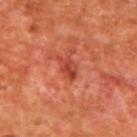No biopsy was performed on this lesion — it was imaged during a full skin examination and was not determined to be concerning. Cropped from a whole-body photographic skin survey; the tile spans about 15 mm. The subject is a male aged 63–67. An algorithmic analysis of the crop reported a shape eccentricity near 0.8. It also reported a nevus-likeness score of about 0/100 and a lesion-detection confidence of about 100/100. The recorded lesion diameter is about 3 mm. The tile uses cross-polarized illumination. The lesion is located on the upper back.